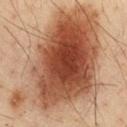{"biopsy_status": "not biopsied; imaged during a skin examination", "automated_metrics": {"area_mm2_approx": 100.0, "shape_asymmetry": 0.3, "cielab_L": 47, "cielab_a": 21, "cielab_b": 31, "vs_skin_darker_L": 16.0, "vs_skin_contrast_norm": 11.0}, "site": "chest", "patient": {"sex": "male", "age_approx": 35}, "image": {"source": "total-body photography crop", "field_of_view_mm": 15}, "lesion_size": {"long_diameter_mm_approx": 15.0}}